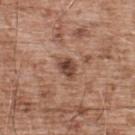Findings:
- follow-up — imaged on a skin check; not biopsied
- imaging modality — 15 mm crop, total-body photography
- subject — male, approximately 55 years of age
- tile lighting — white-light illumination
- site — the upper back
- diameter — ~3 mm (longest diameter)